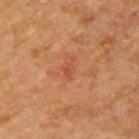The lesion was tiled from a total-body skin photograph and was not biopsied.
Captured under cross-polarized illumination.
A male patient aged 63 to 67.
Automated tile analysis of the lesion measured a border-irregularity rating of about 5.5/10. It also reported a nevus-likeness score of about 5/100 and lesion-presence confidence of about 100/100.
Measured at roughly 2.5 mm in maximum diameter.
Located on the upper back.
A 15 mm close-up extracted from a 3D total-body photography capture.